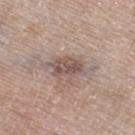Part of a total-body skin-imaging series; this lesion was reviewed on a skin check and was not flagged for biopsy.
The recorded lesion diameter is about 4 mm.
A male subject approximately 80 years of age.
The tile uses white-light illumination.
The lesion is located on the right thigh.
Cropped from a whole-body photographic skin survey; the tile spans about 15 mm.